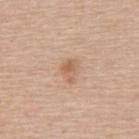notes: total-body-photography surveillance lesion; no biopsy | lighting: white-light | subject: male, roughly 60 years of age | imaging modality: ~15 mm crop, total-body skin-cancer survey | site: the upper back.